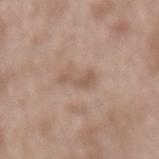workup: no biopsy performed (imaged during a skin exam) | acquisition: ~15 mm tile from a whole-body skin photo | lighting: white-light illumination | size: ≈4 mm | subject: male, approximately 55 years of age | body site: the lower back.